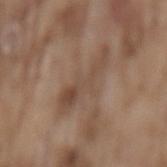Imaged during a routine full-body skin examination; the lesion was not biopsied and no histopathology is available.
A region of skin cropped from a whole-body photographic capture, roughly 15 mm wide.
The lesion is located on the mid back.
A male patient in their mid- to late 70s.
Longest diameter approximately 6.5 mm.
The tile uses white-light illumination.
The lesion-visualizer software estimated an average lesion color of about L≈47 a*≈16 b*≈27 (CIELAB). And it measured an automated nevus-likeness rating near 0 out of 100 and a lesion-detection confidence of about 70/100.